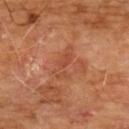Findings:
• workup — no biopsy performed (imaged during a skin exam)
• image-analysis metrics — border irregularity of about 5 on a 0–10 scale and internal color variation of about 2.5 on a 0–10 scale
• illumination — cross-polarized
• lesion diameter — ~4.5 mm (longest diameter)
• site — the chest
• subject — male, aged 58–62
• image source — total-body-photography crop, ~15 mm field of view